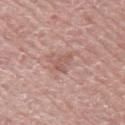Impression: No biopsy was performed on this lesion — it was imaged during a full skin examination and was not determined to be concerning.